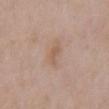Findings:
- biopsy status — catalogued during a skin exam; not biopsied
- image source — ~15 mm tile from a whole-body skin photo
- lesion diameter — ≈2.5 mm
- patient — male, in their mid- to late 60s
- lighting — white-light
- anatomic site — the mid back